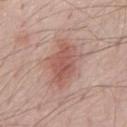biopsy_status: not biopsied; imaged during a skin examination